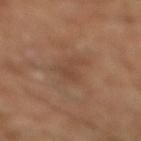Q: Was this lesion biopsied?
A: imaged on a skin check; not biopsied
Q: What lighting was used for the tile?
A: cross-polarized illumination
Q: What are the patient's age and sex?
A: male, aged approximately 65
Q: What kind of image is this?
A: ~15 mm tile from a whole-body skin photo
Q: What is the anatomic site?
A: the right lower leg